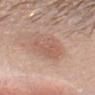Notes:
* biopsy status: no biopsy performed (imaged during a skin exam)
* imaging modality: 15 mm crop, total-body photography
* patient: male, roughly 40 years of age
* site: the head or neck
* lesion size: ~4.5 mm (longest diameter)